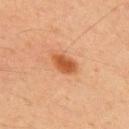biopsy_status: not biopsied; imaged during a skin examination
patient:
  sex: male
  age_approx: 70
lesion_size:
  long_diameter_mm_approx: 3.5
automated_metrics:
  area_mm2_approx: 6.5
  shape_asymmetry: 0.15
  nevus_likeness_0_100: 100
  lesion_detection_confidence_0_100: 100
image:
  source: total-body photography crop
  field_of_view_mm: 15
lighting: cross-polarized
site: chest